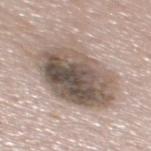{"biopsy_status": "not biopsied; imaged during a skin examination", "automated_metrics": {"area_mm2_approx": 41.0, "shape_asymmetry": 0.15, "nevus_likeness_0_100": 20}, "image": {"source": "total-body photography crop", "field_of_view_mm": 15}, "lesion_size": {"long_diameter_mm_approx": 8.5}, "site": "mid back", "patient": {"sex": "male", "age_approx": 55}}